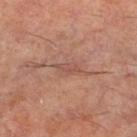{"biopsy_status": "not biopsied; imaged during a skin examination", "lighting": "cross-polarized", "lesion_size": {"long_diameter_mm_approx": 3.0}, "patient": {"sex": "male", "age_approx": 60}, "image": {"source": "total-body photography crop", "field_of_view_mm": 15}, "site": "left lower leg"}